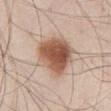Q: Was this lesion biopsied?
A: catalogued during a skin exam; not biopsied
Q: What kind of image is this?
A: ~15 mm crop, total-body skin-cancer survey
Q: Lesion location?
A: the abdomen
Q: Patient demographics?
A: male, aged 48 to 52